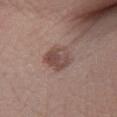Impression: Recorded during total-body skin imaging; not selected for excision or biopsy. Clinical summary: The subject is a male roughly 45 years of age. A roughly 15 mm field-of-view crop from a total-body skin photograph. The lesion is on the right lower leg.